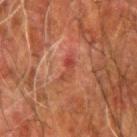Background: Automated image analysis of the tile measured a lesion color around L≈36 a*≈25 b*≈27 in CIELAB, roughly 6 lightness units darker than nearby skin, and a normalized border contrast of about 6. The analysis additionally found a border-irregularity index near 5.5/10. It also reported an automated nevus-likeness rating near 0 out of 100 and a lesion-detection confidence of about 90/100. A region of skin cropped from a whole-body photographic capture, roughly 15 mm wide. The lesion is located on the left forearm. This is a cross-polarized tile. Measured at roughly 3.5 mm in maximum diameter. The patient is a male aged 78–82.